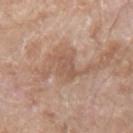Image and clinical context:
The subject is a male aged around 80. A 15 mm crop from a total-body photograph taken for skin-cancer surveillance. The lesion is located on the right forearm. An algorithmic analysis of the crop reported an area of roughly 6 mm² and a symmetry-axis asymmetry near 0.35. It also reported an average lesion color of about L≈55 a*≈19 b*≈28 (CIELAB), about 8 CIELAB-L* units darker than the surrounding skin, and a normalized border contrast of about 5.5. It also reported a border-irregularity index near 5/10. It also reported a classifier nevus-likeness of about 0/100 and lesion-presence confidence of about 80/100. About 4 mm across.